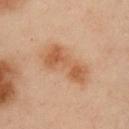| feature | finding |
|---|---|
| biopsy status | no biopsy performed (imaged during a skin exam) |